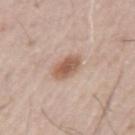Background: The tile uses white-light illumination. A male subject about 55 years old. A region of skin cropped from a whole-body photographic capture, roughly 15 mm wide. About 3.5 mm across. An algorithmic analysis of the crop reported a lesion area of about 6.5 mm². The analysis additionally found a border-irregularity rating of about 2/10 and a color-variation rating of about 4/10.